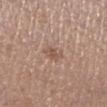workup — imaged on a skin check; not biopsied | acquisition — total-body-photography crop, ~15 mm field of view | site — the left lower leg | size — about 2.5 mm | TBP lesion metrics — an average lesion color of about L≈53 a*≈18 b*≈26 (CIELAB), roughly 8 lightness units darker than nearby skin, and a lesion-to-skin contrast of about 6 (normalized; higher = more distinct); a classifier nevus-likeness of about 0/100 and lesion-presence confidence of about 100/100 | patient — male, aged approximately 65 | illumination — white-light illumination.